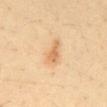Impression:
Recorded during total-body skin imaging; not selected for excision or biopsy.
Image and clinical context:
The patient is a male approximately 35 years of age. Imaged with cross-polarized lighting. Cropped from a whole-body photographic skin survey; the tile spans about 15 mm. The recorded lesion diameter is about 3.5 mm. The lesion-visualizer software estimated a nevus-likeness score of about 85/100 and a lesion-detection confidence of about 100/100. On the abdomen.